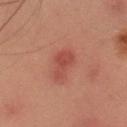Assessment:
Captured during whole-body skin photography for melanoma surveillance; the lesion was not biopsied.
Image and clinical context:
A region of skin cropped from a whole-body photographic capture, roughly 15 mm wide. A female subject approximately 20 years of age. The lesion is located on the head or neck. Imaged with cross-polarized lighting. Longest diameter approximately 3.5 mm.